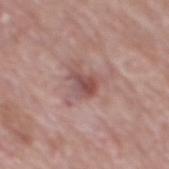No biopsy was performed on this lesion — it was imaged during a full skin examination and was not determined to be concerning.
Longest diameter approximately 3 mm.
A 15 mm close-up extracted from a 3D total-body photography capture.
A male subject aged 68 to 72.
Automated tile analysis of the lesion measured an eccentricity of roughly 0.7 and two-axis asymmetry of about 0.4. It also reported a lesion color around L≈49 a*≈23 b*≈21 in CIELAB, about 11 CIELAB-L* units darker than the surrounding skin, and a normalized border contrast of about 7.5. The software also gave a within-lesion color-variation index near 3.5/10 and peripheral color asymmetry of about 1. The analysis additionally found a nevus-likeness score of about 0/100 and lesion-presence confidence of about 100/100.
This is a white-light tile.
Located on the mid back.